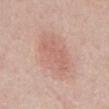Q: Lesion size?
A: ≈6.5 mm
Q: How was the tile lit?
A: white-light illumination
Q: What did automated image analysis measure?
A: an average lesion color of about L≈63 a*≈21 b*≈26 (CIELAB), a lesion–skin lightness drop of about 7, and a normalized lesion–skin contrast near 4.5
Q: How was this image acquired?
A: ~15 mm crop, total-body skin-cancer survey
Q: Where on the body is the lesion?
A: the front of the torso
Q: Who is the patient?
A: male, aged around 70